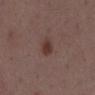Impression:
The lesion was tiled from a total-body skin photograph and was not biopsied.
Context:
The subject is a male aged 48 to 52. About 3 mm across. Cropped from a total-body skin-imaging series; the visible field is about 15 mm. On the mid back. Imaged with white-light lighting.